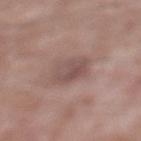Imaged during a routine full-body skin examination; the lesion was not biopsied and no histopathology is available. A male patient, roughly 60 years of age. The lesion is on the right lower leg. Captured under white-light illumination. This image is a 15 mm lesion crop taken from a total-body photograph.